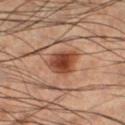Q: Was a biopsy performed?
A: total-body-photography surveillance lesion; no biopsy
Q: Lesion location?
A: the right lower leg
Q: What is the lesion's diameter?
A: about 3.5 mm
Q: What are the patient's age and sex?
A: male, aged 48–52
Q: What lighting was used for the tile?
A: cross-polarized illumination
Q: How was this image acquired?
A: ~15 mm tile from a whole-body skin photo